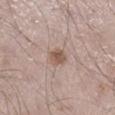| feature | finding |
|---|---|
| follow-up | no biopsy performed (imaged during a skin exam) |
| acquisition | ~15 mm tile from a whole-body skin photo |
| anatomic site | the leg |
| subject | male, aged 58 to 62 |